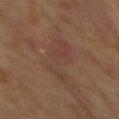Imaged during a routine full-body skin examination; the lesion was not biopsied and no histopathology is available.
The subject is a male in their 70s.
From the mid back.
Cropped from a total-body skin-imaging series; the visible field is about 15 mm.